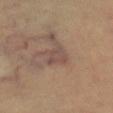<record>
<biopsy_status>not biopsied; imaged during a skin examination</biopsy_status>
<lesion_size>
  <long_diameter_mm_approx>3.0</long_diameter_mm_approx>
</lesion_size>
<patient>
  <age_approx>60</age_approx>
</patient>
<image>
  <source>total-body photography crop</source>
  <field_of_view_mm>15</field_of_view_mm>
</image>
<site>left thigh</site>
</record>